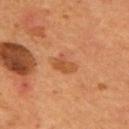<record>
  <biopsy_status>not biopsied; imaged during a skin examination</biopsy_status>
  <automated_metrics>
    <border_irregularity_0_10>3.0</border_irregularity_0_10>
    <peripheral_color_asymmetry>1.0</peripheral_color_asymmetry>
    <nevus_likeness_0_100>15</nevus_likeness_0_100>
    <lesion_detection_confidence_0_100>100</lesion_detection_confidence_0_100>
  </automated_metrics>
  <lighting>cross-polarized</lighting>
  <site>upper back</site>
  <image>
    <source>total-body photography crop</source>
    <field_of_view_mm>15</field_of_view_mm>
  </image>
  <patient>
    <sex>male</sex>
    <age_approx>55</age_approx>
  </patient>
  <lesion_size>
    <long_diameter_mm_approx>2.5</long_diameter_mm_approx>
  </lesion_size>
</record>